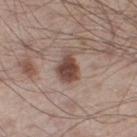| feature | finding |
|---|---|
| biopsy status | no biopsy performed (imaged during a skin exam) |
| TBP lesion metrics | a border-irregularity index near 2.5/10, internal color variation of about 3 on a 0–10 scale, and radial color variation of about 1; a nevus-likeness score of about 95/100 and a lesion-detection confidence of about 100/100 |
| site | the right thigh |
| subject | male, in their mid-50s |
| imaging modality | ~15 mm tile from a whole-body skin photo |
| lesion diameter | ≈3 mm |